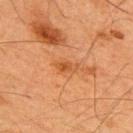No biopsy was performed on this lesion — it was imaged during a full skin examination and was not determined to be concerning. The lesion is on the upper back. A male subject, aged 63–67. The recorded lesion diameter is about 2.5 mm. The total-body-photography lesion software estimated a lesion color around L≈44 a*≈24 b*≈37 in CIELAB, roughly 7 lightness units darker than nearby skin, and a lesion-to-skin contrast of about 6.5 (normalized; higher = more distinct). And it measured a border-irregularity index near 3.5/10. A 15 mm crop from a total-body photograph taken for skin-cancer surveillance.